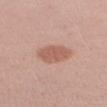A female subject in their 30s.
Longest diameter approximately 4 mm.
The lesion is on the right upper arm.
Automated tile analysis of the lesion measured a lesion color around L≈58 a*≈23 b*≈27 in CIELAB, about 10 CIELAB-L* units darker than the surrounding skin, and a normalized lesion–skin contrast near 6.5.
The tile uses white-light illumination.
A region of skin cropped from a whole-body photographic capture, roughly 15 mm wide.